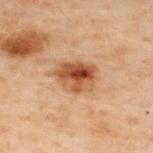A male patient aged 48 to 52. Cropped from a whole-body photographic skin survey; the tile spans about 15 mm. The lesion is located on the upper back.